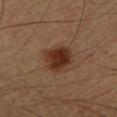Part of a total-body skin-imaging series; this lesion was reviewed on a skin check and was not flagged for biopsy. Automated tile analysis of the lesion measured a nevus-likeness score of about 95/100 and lesion-presence confidence of about 100/100. A 15 mm close-up tile from a total-body photography series done for melanoma screening. A male patient aged approximately 55. Imaged with cross-polarized lighting. The lesion is located on the left forearm. Approximately 3.5 mm at its widest.